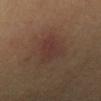Imaged with cross-polarized lighting. Approximately 4.5 mm at its widest. A female subject, aged around 55. The lesion is on the left forearm. A close-up tile cropped from a whole-body skin photograph, about 15 mm across. The lesion-visualizer software estimated a lesion area of about 11 mm², a shape eccentricity near 0.7, and a shape-asymmetry score of about 0.35 (0 = symmetric). It also reported a border-irregularity index near 3.5/10, internal color variation of about 4 on a 0–10 scale, and peripheral color asymmetry of about 1.5.